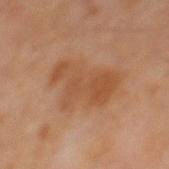Recorded during total-body skin imaging; not selected for excision or biopsy. Longest diameter approximately 6.5 mm. The patient is a male in their 60s. A 15 mm close-up tile from a total-body photography series done for melanoma screening. The lesion-visualizer software estimated a footprint of about 22 mm², a shape eccentricity near 0.7, and two-axis asymmetry of about 0.35. The software also gave an automated nevus-likeness rating near 5 out of 100 and lesion-presence confidence of about 100/100. This is a cross-polarized tile. From the mid back.